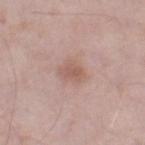Assessment:
The lesion was tiled from a total-body skin photograph and was not biopsied.
Acquisition and patient details:
A region of skin cropped from a whole-body photographic capture, roughly 15 mm wide. Located on the right thigh. Automated image analysis of the tile measured a lesion area of about 5 mm², a shape eccentricity near 0.8, and a symmetry-axis asymmetry near 0.25. The software also gave a mean CIELAB color near L≈57 a*≈20 b*≈25 and a lesion-to-skin contrast of about 6 (normalized; higher = more distinct). A male patient in their mid- to late 50s. The recorded lesion diameter is about 3 mm.